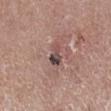The lesion was tiled from a total-body skin photograph and was not biopsied. Automated tile analysis of the lesion measured an area of roughly 4.5 mm² and a shape eccentricity near 0.85. And it measured a mean CIELAB color near L≈47 a*≈17 b*≈18 and about 12 CIELAB-L* units darker than the surrounding skin. It also reported lesion-presence confidence of about 95/100. Approximately 3 mm at its widest. A male patient aged approximately 50. Cropped from a total-body skin-imaging series; the visible field is about 15 mm. Captured under white-light illumination. On the right lower leg.